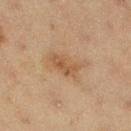{
  "biopsy_status": "not biopsied; imaged during a skin examination",
  "patient": {
    "sex": "female",
    "age_approx": 50
  },
  "site": "leg",
  "lesion_size": {
    "long_diameter_mm_approx": 5.0
  },
  "lighting": "cross-polarized",
  "image": {
    "source": "total-body photography crop",
    "field_of_view_mm": 15
  }
}